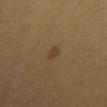biopsy_status: not biopsied; imaged during a skin examination
lighting: cross-polarized
lesion_size:
  long_diameter_mm_approx: 2.5
patient:
  sex: female
  age_approx: 30
image:
  source: total-body photography crop
  field_of_view_mm: 15
site: mid back